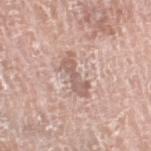Impression:
No biopsy was performed on this lesion — it was imaged during a full skin examination and was not determined to be concerning.
Acquisition and patient details:
Located on the right lower leg. A male patient, roughly 75 years of age. A 15 mm close-up tile from a total-body photography series done for melanoma screening.